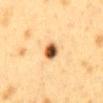Impression:
The lesion was tiled from a total-body skin photograph and was not biopsied.
Background:
This is a cross-polarized tile. A region of skin cropped from a whole-body photographic capture, roughly 15 mm wide. The lesion is located on the mid back. The lesion's longest dimension is about 3 mm. A female patient, aged approximately 40. The total-body-photography lesion software estimated a mean CIELAB color near L≈48 a*≈19 b*≈36, a lesion–skin lightness drop of about 20, and a normalized border contrast of about 13.5. The software also gave a classifier nevus-likeness of about 100/100.